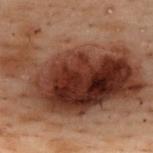An algorithmic analysis of the crop reported a footprint of about 100 mm².
A female patient, approximately 55 years of age.
A lesion tile, about 15 mm wide, cut from a 3D total-body photograph.
On the upper back.